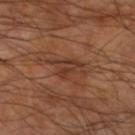Cropped from a whole-body photographic skin survey; the tile spans about 15 mm. Measured at roughly 4.5 mm in maximum diameter. A male patient approximately 60 years of age. From the leg.